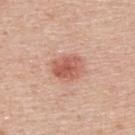Imaged during a routine full-body skin examination; the lesion was not biopsied and no histopathology is available.
Cropped from a whole-body photographic skin survey; the tile spans about 15 mm.
A male subject aged 38 to 42.
The lesion is located on the back.
The lesion's longest dimension is about 3.5 mm.
The lesion-visualizer software estimated a footprint of about 8.5 mm², an outline eccentricity of about 0.65 (0 = round, 1 = elongated), and a symmetry-axis asymmetry near 0.15. It also reported a within-lesion color-variation index near 3.5/10 and radial color variation of about 1.